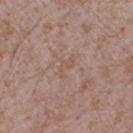Notes:
– follow-up: total-body-photography surveillance lesion; no biopsy
– diameter: about 3 mm
– acquisition: ~15 mm tile from a whole-body skin photo
– subject: male, in their mid-70s
– site: the right forearm
– lighting: white-light